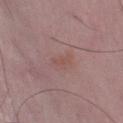This lesion was catalogued during total-body skin photography and was not selected for biopsy. Imaged with white-light lighting. Cropped from a total-body skin-imaging series; the visible field is about 15 mm. The patient is a male aged around 50. From the leg.